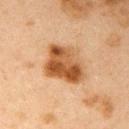Clinical impression:
Captured during whole-body skin photography for melanoma surveillance; the lesion was not biopsied.
Context:
Measured at roughly 5 mm in maximum diameter. Located on the right upper arm. Cropped from a total-body skin-imaging series; the visible field is about 15 mm. The subject is a female aged 38 to 42. This is a cross-polarized tile.